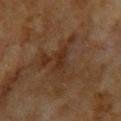Imaged during a routine full-body skin examination; the lesion was not biopsied and no histopathology is available. The lesion is located on the upper back. A lesion tile, about 15 mm wide, cut from a 3D total-body photograph. A female subject, aged 58 to 62.